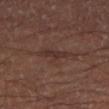Imaged during a routine full-body skin examination; the lesion was not biopsied and no histopathology is available. A male subject in their mid- to late 50s. Automated tile analysis of the lesion measured an area of roughly 4 mm² and an outline eccentricity of about 0.9 (0 = round, 1 = elongated). It also reported a border-irregularity index near 3/10 and a peripheral color-asymmetry measure near 0. And it measured lesion-presence confidence of about 75/100. The lesion's longest dimension is about 3.5 mm. A close-up tile cropped from a whole-body skin photograph, about 15 mm across. On the right thigh. This is a cross-polarized tile.